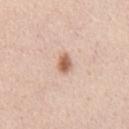  biopsy_status: not biopsied; imaged during a skin examination
  site: chest
  lesion_size:
    long_diameter_mm_approx: 2.5
  lighting: white-light
  patient:
    sex: male
    age_approx: 45
  image:
    source: total-body photography crop
    field_of_view_mm: 15
  automated_metrics:
    nevus_likeness_0_100: 95
    lesion_detection_confidence_0_100: 100